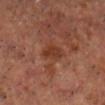Assessment: Recorded during total-body skin imaging; not selected for excision or biopsy. Image and clinical context: A 15 mm close-up tile from a total-body photography series done for melanoma screening. Automated tile analysis of the lesion measured a footprint of about 5.5 mm², a shape eccentricity near 0.5, and a shape-asymmetry score of about 0.25 (0 = symmetric). And it measured about 7 CIELAB-L* units darker than the surrounding skin and a lesion-to-skin contrast of about 7 (normalized; higher = more distinct). The software also gave a detector confidence of about 100 out of 100 that the crop contains a lesion. This is a cross-polarized tile. On the head or neck. About 3 mm across. A male patient roughly 65 years of age.